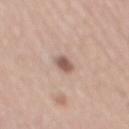biopsy_status: not biopsied; imaged during a skin examination
image:
  source: total-body photography crop
  field_of_view_mm: 15
lesion_size:
  long_diameter_mm_approx: 3.0
patient:
  sex: male
  age_approx: 65
site: mid back
automated_metrics:
  eccentricity: 0.85
  shape_asymmetry: 0.2
  vs_skin_darker_L: 13.0
  vs_skin_contrast_norm: 8.5
  border_irregularity_0_10: 2.0
  color_variation_0_10: 3.0
  peripheral_color_asymmetry: 1.0